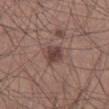Part of a total-body skin-imaging series; this lesion was reviewed on a skin check and was not flagged for biopsy.